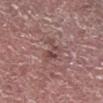Impression: The lesion was tiled from a total-body skin photograph and was not biopsied. Context: A 15 mm close-up extracted from a 3D total-body photography capture. On the right lower leg. This is a white-light tile. An algorithmic analysis of the crop reported a mean CIELAB color near L≈46 a*≈20 b*≈20 and a lesion–skin lightness drop of about 8. And it measured peripheral color asymmetry of about 2.5. The software also gave a classifier nevus-likeness of about 0/100. Measured at roughly 3.5 mm in maximum diameter. A male subject in their mid- to late 70s.